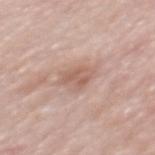This lesion was catalogued during total-body skin photography and was not selected for biopsy. A male subject aged 53 to 57. The tile uses white-light illumination. The recorded lesion diameter is about 3.5 mm. From the mid back. A 15 mm close-up tile from a total-body photography series done for melanoma screening.